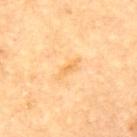Clinical impression: Recorded during total-body skin imaging; not selected for excision or biopsy. Context: This image is a 15 mm lesion crop taken from a total-body photograph. About 3.5 mm across. A male patient, approximately 85 years of age or older. The tile uses cross-polarized illumination. Automated tile analysis of the lesion measured a footprint of about 3 mm² and two-axis asymmetry of about 0.35. It also reported a normalized border contrast of about 6. And it measured a border-irregularity index near 4/10. Located on the upper back.